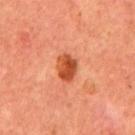Imaged during a routine full-body skin examination; the lesion was not biopsied and no histopathology is available.
Imaged with cross-polarized lighting.
An algorithmic analysis of the crop reported a mean CIELAB color near L≈46 a*≈32 b*≈38 and roughly 13 lightness units darker than nearby skin. It also reported lesion-presence confidence of about 100/100.
The patient is a male aged 63 to 67.
Located on the chest.
Cropped from a whole-body photographic skin survey; the tile spans about 15 mm.
The recorded lesion diameter is about 3.5 mm.